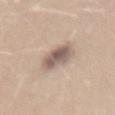No biopsy was performed on this lesion — it was imaged during a full skin examination and was not determined to be concerning.
This is a white-light tile.
Measured at roughly 4 mm in maximum diameter.
A female patient, about 25 years old.
Automated tile analysis of the lesion measured a mean CIELAB color near L≈57 a*≈15 b*≈22, roughly 14 lightness units darker than nearby skin, and a lesion-to-skin contrast of about 9.5 (normalized; higher = more distinct). It also reported a border-irregularity rating of about 2/10. It also reported an automated nevus-likeness rating near 10 out of 100 and a lesion-detection confidence of about 100/100.
A region of skin cropped from a whole-body photographic capture, roughly 15 mm wide.
From the mid back.